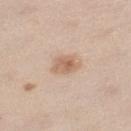biopsy_status: not biopsied; imaged during a skin examination
image:
  source: total-body photography crop
  field_of_view_mm: 15
lesion_size:
  long_diameter_mm_approx: 3.0
site: leg
lighting: white-light
patient:
  sex: female
  age_approx: 40